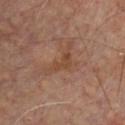Part of a total-body skin-imaging series; this lesion was reviewed on a skin check and was not flagged for biopsy. Measured at roughly 3 mm in maximum diameter. Captured under cross-polarized illumination. From the chest. A male patient, aged around 60. A 15 mm close-up tile from a total-body photography series done for melanoma screening. An algorithmic analysis of the crop reported a footprint of about 4 mm², an outline eccentricity of about 0.85 (0 = round, 1 = elongated), and a shape-asymmetry score of about 0.55 (0 = symmetric). The analysis additionally found a border-irregularity rating of about 5.5/10 and a within-lesion color-variation index near 2.5/10.